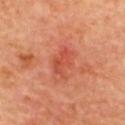The lesion was photographed on a routine skin check and not biopsied; there is no pathology result.
About 3.5 mm across.
On the upper back.
A male subject, roughly 60 years of age.
Captured under cross-polarized illumination.
Cropped from a total-body skin-imaging series; the visible field is about 15 mm.